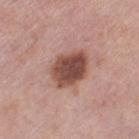Captured during whole-body skin photography for melanoma surveillance; the lesion was not biopsied.